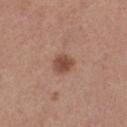follow-up=imaged on a skin check; not biopsied
site=the leg
patient=female, aged 43 to 47
lighting=white-light illumination
lesion diameter=~2.5 mm (longest diameter)
image source=total-body-photography crop, ~15 mm field of view